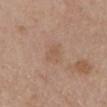Cropped from a total-body skin-imaging series; the visible field is about 15 mm.
On the abdomen.
A male patient aged approximately 80.